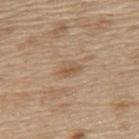Q: What are the patient's age and sex?
A: male, aged around 70
Q: How large is the lesion?
A: ≈3 mm
Q: What kind of image is this?
A: total-body-photography crop, ~15 mm field of view
Q: Where on the body is the lesion?
A: the upper back
Q: How was the tile lit?
A: white-light